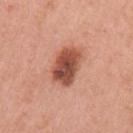Case summary:
– workup — no biopsy performed (imaged during a skin exam)
– illumination — white-light
– automated metrics — a shape eccentricity near 0.7
– patient — female, roughly 55 years of age
– imaging modality — ~15 mm tile from a whole-body skin photo
– size — ≈4.5 mm
– location — the left upper arm